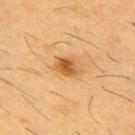Recorded during total-body skin imaging; not selected for excision or biopsy.
Imaged with cross-polarized lighting.
A male subject, aged around 55.
The recorded lesion diameter is about 3 mm.
A lesion tile, about 15 mm wide, cut from a 3D total-body photograph.
The total-body-photography lesion software estimated an area of roughly 4.5 mm², a shape eccentricity near 0.75, and two-axis asymmetry of about 0.2. The analysis additionally found lesion-presence confidence of about 100/100.
The lesion is on the chest.